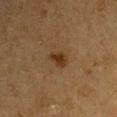workup: imaged on a skin check; not biopsied.